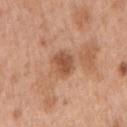The lesion was tiled from a total-body skin photograph and was not biopsied.
This is a white-light tile.
An algorithmic analysis of the crop reported a mean CIELAB color near L≈52 a*≈24 b*≈33, a lesion–skin lightness drop of about 11, and a normalized lesion–skin contrast near 7.5. And it measured a border-irregularity rating of about 2/10 and radial color variation of about 0.5.
The lesion is on the right upper arm.
A roughly 15 mm field-of-view crop from a total-body skin photograph.
The subject is a female approximately 40 years of age.